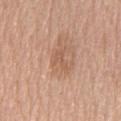– workup: no biopsy performed (imaged during a skin exam)
– diameter: ≈3 mm
– anatomic site: the front of the torso
– acquisition: ~15 mm crop, total-body skin-cancer survey
– patient: male, aged 78–82
– TBP lesion metrics: a border-irregularity rating of about 7/10, a within-lesion color-variation index near 0/10, and a peripheral color-asymmetry measure near 0; a nevus-likeness score of about 0/100 and lesion-presence confidence of about 100/100